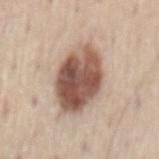biopsy status = no biopsy performed (imaged during a skin exam)
body site = the back
patient = male, about 60 years old
acquisition = ~15 mm tile from a whole-body skin photo